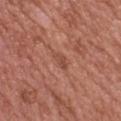{
  "site": "upper back",
  "lighting": "white-light",
  "patient": {
    "sex": "female",
    "age_approx": 60
  },
  "automated_metrics": {
    "eccentricity": 0.9,
    "border_irregularity_0_10": 3.5,
    "peripheral_color_asymmetry": 0.5,
    "lesion_detection_confidence_0_100": 100
  },
  "image": {
    "source": "total-body photography crop",
    "field_of_view_mm": 15
  }
}